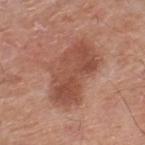The lesion was photographed on a routine skin check and not biopsied; there is no pathology result. This is a white-light tile. A region of skin cropped from a whole-body photographic capture, roughly 15 mm wide. Located on the right lower leg. An algorithmic analysis of the crop reported an average lesion color of about L≈50 a*≈24 b*≈29 (CIELAB), a lesion–skin lightness drop of about 10, and a lesion-to-skin contrast of about 7 (normalized; higher = more distinct). It also reported a border-irregularity rating of about 3.5/10. A male subject, aged 78 to 82.